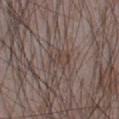<record>
  <biopsy_status>not biopsied; imaged during a skin examination</biopsy_status>
  <image>
    <source>total-body photography crop</source>
    <field_of_view_mm>15</field_of_view_mm>
  </image>
  <site>abdomen</site>
  <patient>
    <sex>male</sex>
    <age_approx>75</age_approx>
  </patient>
</record>